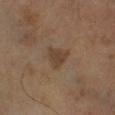Part of a total-body skin-imaging series; this lesion was reviewed on a skin check and was not flagged for biopsy. About 3 mm across. The tile uses cross-polarized illumination. The total-body-photography lesion software estimated an area of roughly 5 mm², a shape eccentricity near 0.55, and two-axis asymmetry of about 0.35. And it measured a border-irregularity rating of about 3.5/10 and a within-lesion color-variation index near 1.5/10. A close-up tile cropped from a whole-body skin photograph, about 15 mm across. A male subject approximately 65 years of age. Located on the left leg.